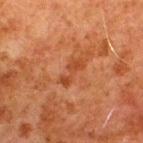Q: Was a biopsy performed?
A: catalogued during a skin exam; not biopsied
Q: How was this image acquired?
A: 15 mm crop, total-body photography
Q: Patient demographics?
A: male, aged around 80
Q: Lesion size?
A: ~3.5 mm (longest diameter)
Q: What lighting was used for the tile?
A: cross-polarized
Q: Lesion location?
A: the upper back